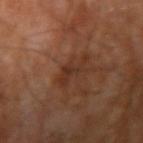Assessment:
This lesion was catalogued during total-body skin photography and was not selected for biopsy.
Clinical summary:
From the right upper arm. Imaged with cross-polarized lighting. A lesion tile, about 15 mm wide, cut from a 3D total-body photograph. The subject is a male aged 68 to 72. Measured at roughly 4 mm in maximum diameter.